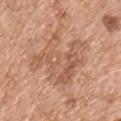Clinical impression: This lesion was catalogued during total-body skin photography and was not selected for biopsy. Background: Automated image analysis of the tile measured an eccentricity of roughly 0.7 and two-axis asymmetry of about 0.5. The software also gave a mean CIELAB color near L≈58 a*≈22 b*≈32. The software also gave border irregularity of about 8.5 on a 0–10 scale and internal color variation of about 4.5 on a 0–10 scale. The software also gave a classifier nevus-likeness of about 0/100 and a lesion-detection confidence of about 95/100. Cropped from a total-body skin-imaging series; the visible field is about 15 mm. From the upper back. The patient is a male aged around 75. The tile uses white-light illumination.